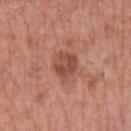Imaged during a routine full-body skin examination; the lesion was not biopsied and no histopathology is available. A 15 mm close-up tile from a total-body photography series done for melanoma screening. The tile uses white-light illumination. The patient is a male aged 78 to 82. An algorithmic analysis of the crop reported an area of roughly 7 mm² and an eccentricity of roughly 0.55. The software also gave a mean CIELAB color near L≈49 a*≈25 b*≈29 and a lesion-to-skin contrast of about 7.5 (normalized; higher = more distinct). The analysis additionally found lesion-presence confidence of about 100/100. From the left lower leg. The recorded lesion diameter is about 3 mm.